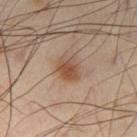Imaged during a routine full-body skin examination; the lesion was not biopsied and no histopathology is available. The lesion is on the left thigh. The tile uses cross-polarized illumination. A male patient in their mid-50s. This image is a 15 mm lesion crop taken from a total-body photograph.